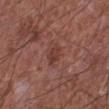  biopsy_status: not biopsied; imaged during a skin examination
  automated_metrics:
    cielab_L: 38
    cielab_a: 23
    cielab_b: 23
    vs_skin_darker_L: 7.0
    vs_skin_contrast_norm: 6.5
    border_irregularity_0_10: 2.5
    color_variation_0_10: 2.0
    peripheral_color_asymmetry: 1.0
  site: left lower leg
  lesion_size:
    long_diameter_mm_approx: 2.5
  image:
    source: total-body photography crop
    field_of_view_mm: 15
  patient:
    sex: male
    age_approx: 65